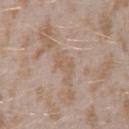Recorded during total-body skin imaging; not selected for excision or biopsy. Located on the left forearm. A female subject about 25 years old. A 15 mm close-up tile from a total-body photography series done for melanoma screening.